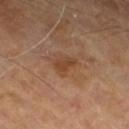Assessment: The lesion was photographed on a routine skin check and not biopsied; there is no pathology result. Context: Automated image analysis of the tile measured a border-irregularity rating of about 4/10 and radial color variation of about 0.5. A subject in their mid-60s. This is a cross-polarized tile. The lesion is located on the right thigh. Cropped from a total-body skin-imaging series; the visible field is about 15 mm. Approximately 3 mm at its widest.